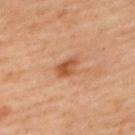follow-up — imaged on a skin check; not biopsied
imaging modality — ~15 mm crop, total-body skin-cancer survey
subject — female, roughly 40 years of age
size — ~3 mm (longest diameter)
illumination — cross-polarized illumination
site — the upper back